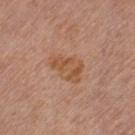Part of a total-body skin-imaging series; this lesion was reviewed on a skin check and was not flagged for biopsy. The subject is a female in their mid-50s. Longest diameter approximately 4.5 mm. Located on the left thigh. This is a white-light tile. Cropped from a total-body skin-imaging series; the visible field is about 15 mm.